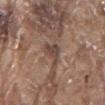| key | value |
|---|---|
| workup | catalogued during a skin exam; not biopsied |
| anatomic site | the mid back |
| lighting | white-light illumination |
| patient | male, in their 80s |
| size | ≈3.5 mm |
| automated lesion analysis | an area of roughly 5 mm², a shape eccentricity near 0.75, and a symmetry-axis asymmetry near 0.5; a within-lesion color-variation index near 3/10 |
| image | ~15 mm tile from a whole-body skin photo |